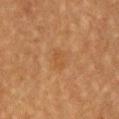Recorded during total-body skin imaging; not selected for excision or biopsy. Measured at roughly 2.5 mm in maximum diameter. Automated image analysis of the tile measured border irregularity of about 3 on a 0–10 scale, a color-variation rating of about 1.5/10, and peripheral color asymmetry of about 0.5. It also reported a nevus-likeness score of about 5/100. The tile uses cross-polarized illumination. A male subject, in their mid-60s. A region of skin cropped from a whole-body photographic capture, roughly 15 mm wide. From the chest.